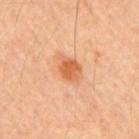<case>
  <biopsy_status>not biopsied; imaged during a skin examination</biopsy_status>
  <patient>
    <sex>male</sex>
    <age_approx>60</age_approx>
  </patient>
  <image>
    <source>total-body photography crop</source>
    <field_of_view_mm>15</field_of_view_mm>
  </image>
  <lesion_size>
    <long_diameter_mm_approx>3.0</long_diameter_mm_approx>
  </lesion_size>
  <site>right upper arm</site>
  <lighting>cross-polarized</lighting>
</case>